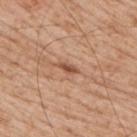– location — the right upper arm
– image — ~15 mm tile from a whole-body skin photo
– lesion diameter — about 2.5 mm
– patient — male, aged approximately 65
– lighting — white-light illumination